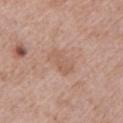The lesion's longest dimension is about 3.5 mm.
Cropped from a total-body skin-imaging series; the visible field is about 15 mm.
The total-body-photography lesion software estimated a border-irregularity index near 2/10, a within-lesion color-variation index near 3/10, and a peripheral color-asymmetry measure near 1. The software also gave a classifier nevus-likeness of about 0/100 and lesion-presence confidence of about 100/100.
From the left upper arm.
A male patient, about 65 years old.
This is a white-light tile.